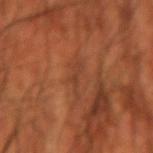Impression: No biopsy was performed on this lesion — it was imaged during a full skin examination and was not determined to be concerning. Clinical summary: The lesion's longest dimension is about 4 mm. A male subject roughly 70 years of age. Captured under cross-polarized illumination. The lesion is located on the left forearm. A 15 mm crop from a total-body photograph taken for skin-cancer surveillance. Automated image analysis of the tile measured an area of roughly 4 mm² and an outline eccentricity of about 0.9 (0 = round, 1 = elongated). It also reported a within-lesion color-variation index near 0.5/10 and peripheral color asymmetry of about 0. The analysis additionally found a nevus-likeness score of about 0/100 and a lesion-detection confidence of about 55/100.